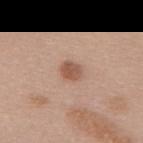subject: female, roughly 60 years of age
lighting: white-light illumination
acquisition: ~15 mm tile from a whole-body skin photo
automated metrics: a lesion area of about 4.5 mm², a shape eccentricity near 0.6, and a symmetry-axis asymmetry near 0.15; an average lesion color of about L≈55 a*≈20 b*≈29 (CIELAB), about 11 CIELAB-L* units darker than the surrounding skin, and a normalized lesion–skin contrast near 7.5; internal color variation of about 2.5 on a 0–10 scale and peripheral color asymmetry of about 1
location: the upper back
lesion diameter: ~2.5 mm (longest diameter)